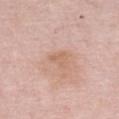The lesion was tiled from a total-body skin photograph and was not biopsied.
Imaged with white-light lighting.
The lesion-visualizer software estimated a lesion area of about 4 mm², an eccentricity of roughly 0.7, and a symmetry-axis asymmetry near 0.35. The software also gave a lesion color around L≈63 a*≈20 b*≈30 in CIELAB and a normalized lesion–skin contrast near 5.
A lesion tile, about 15 mm wide, cut from a 3D total-body photograph.
From the front of the torso.
About 2.5 mm across.
A female subject aged 63 to 67.